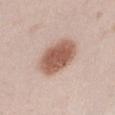Clinical impression:
Imaged during a routine full-body skin examination; the lesion was not biopsied and no histopathology is available.
Context:
Cropped from a total-body skin-imaging series; the visible field is about 15 mm. A female subject, aged 23 to 27. From the right thigh.